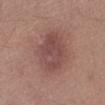notes: total-body-photography surveillance lesion; no biopsy
image source: ~15 mm crop, total-body skin-cancer survey
location: the left lower leg
lesion size: about 5.5 mm
patient: male, about 45 years old
illumination: white-light